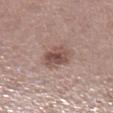  biopsy_status: not biopsied; imaged during a skin examination
  site: leg
  image:
    source: total-body photography crop
    field_of_view_mm: 15
  automated_metrics:
    border_irregularity_0_10: 2.5
    peripheral_color_asymmetry: 1.5
    nevus_likeness_0_100: 30
    lesion_detection_confidence_0_100: 100
  patient:
    sex: female
    age_approx: 50